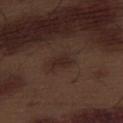No biopsy was performed on this lesion — it was imaged during a full skin examination and was not determined to be concerning. The lesion-visualizer software estimated a footprint of about 4 mm², a shape eccentricity near 0.85, and a shape-asymmetry score of about 0.35 (0 = symmetric). It also reported an average lesion color of about L≈25 a*≈16 b*≈20 (CIELAB), roughly 5 lightness units darker than nearby skin, and a lesion-to-skin contrast of about 6 (normalized; higher = more distinct). It also reported a lesion-detection confidence of about 100/100. The recorded lesion diameter is about 3 mm. Cropped from a whole-body photographic skin survey; the tile spans about 15 mm. A male patient in their 70s. Captured under white-light illumination. The lesion is on the abdomen.